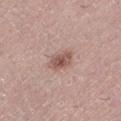No biopsy was performed on this lesion — it was imaged during a full skin examination and was not determined to be concerning. A female patient, roughly 40 years of age. Cropped from a whole-body photographic skin survey; the tile spans about 15 mm. The lesion is on the leg.